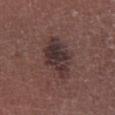Clinical impression:
Imaged during a routine full-body skin examination; the lesion was not biopsied and no histopathology is available.
Acquisition and patient details:
The lesion is located on the right lower leg. Automated tile analysis of the lesion measured an average lesion color of about L≈32 a*≈16 b*≈16 (CIELAB), roughly 9 lightness units darker than nearby skin, and a lesion-to-skin contrast of about 9.5 (normalized; higher = more distinct). The software also gave an automated nevus-likeness rating near 20 out of 100 and lesion-presence confidence of about 100/100. The subject is a male aged 63–67. A roughly 15 mm field-of-view crop from a total-body skin photograph. The lesion's longest dimension is about 5 mm.